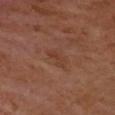Clinical impression:
The lesion was tiled from a total-body skin photograph and was not biopsied.
Image and clinical context:
This is a cross-polarized tile. A lesion tile, about 15 mm wide, cut from a 3D total-body photograph. Measured at roughly 3 mm in maximum diameter. On the left upper arm. A female subject, aged approximately 55.